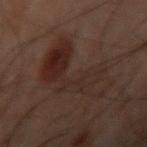Impression: No biopsy was performed on this lesion — it was imaged during a full skin examination and was not determined to be concerning. Clinical summary: Imaged with cross-polarized lighting. On the arm. Approximately 10 mm at its widest. A 15 mm close-up extracted from a 3D total-body photography capture. A male patient about 50 years old.